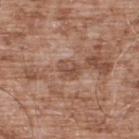Longest diameter approximately 2.5 mm. Imaged with white-light lighting. A male subject aged 53 to 57. Cropped from a total-body skin-imaging series; the visible field is about 15 mm. The lesion-visualizer software estimated a footprint of about 4.5 mm² and two-axis asymmetry of about 0.25. The lesion is located on the back.